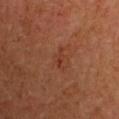Q: Was this lesion biopsied?
A: total-body-photography surveillance lesion; no biopsy
Q: What are the patient's age and sex?
A: male, aged 58 to 62
Q: What is the imaging modality?
A: 15 mm crop, total-body photography
Q: What is the anatomic site?
A: the right upper arm
Q: Lesion size?
A: ≈3 mm